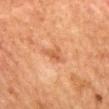{"biopsy_status": "not biopsied; imaged during a skin examination", "image": {"source": "total-body photography crop", "field_of_view_mm": 15}, "automated_metrics": {"eccentricity": 0.85, "peripheral_color_asymmetry": 1.0}, "lighting": "cross-polarized", "site": "mid back", "patient": {"sex": "female", "age_approx": 60}}